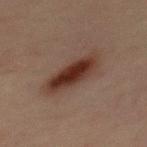This lesion was catalogued during total-body skin photography and was not selected for biopsy. The tile uses cross-polarized illumination. A region of skin cropped from a whole-body photographic capture, roughly 15 mm wide. The lesion is on the mid back. An algorithmic analysis of the crop reported a footprint of about 13 mm² and an eccentricity of roughly 0.85. The software also gave a mean CIELAB color near L≈27 a*≈17 b*≈21, about 11 CIELAB-L* units darker than the surrounding skin, and a normalized border contrast of about 11.5. And it measured a border-irregularity rating of about 2.5/10, a color-variation rating of about 5/10, and radial color variation of about 1.5. The analysis additionally found a classifier nevus-likeness of about 100/100 and a detector confidence of about 100 out of 100 that the crop contains a lesion. A male subject in their 30s. Longest diameter approximately 5.5 mm.